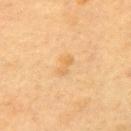follow-up: catalogued during a skin exam; not biopsied | site: the upper back | patient: female, aged around 60 | size: ~2.5 mm (longest diameter) | automated metrics: a lesion area of about 2.5 mm², a shape eccentricity near 0.9, and a symmetry-axis asymmetry near 0.35; a lesion color around L≈61 a*≈17 b*≈41 in CIELAB | acquisition: ~15 mm tile from a whole-body skin photo.